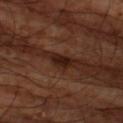Clinical impression:
The lesion was photographed on a routine skin check and not biopsied; there is no pathology result.